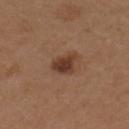follow-up: imaged on a skin check; not biopsied
image source: ~15 mm tile from a whole-body skin photo
anatomic site: the right upper arm
diameter: ~3 mm (longest diameter)
subject: female, in their 40s
lighting: white-light